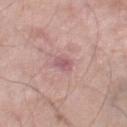Case summary:
– notes — catalogued during a skin exam; not biopsied
– location — the right thigh
– subject — male, aged approximately 70
– imaging modality — ~15 mm crop, total-body skin-cancer survey
– diameter — ≈2.5 mm